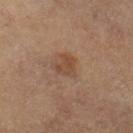{
  "biopsy_status": "not biopsied; imaged during a skin examination",
  "lesion_size": {
    "long_diameter_mm_approx": 2.5
  },
  "patient": {
    "sex": "male",
    "age_approx": 70
  },
  "image": {
    "source": "total-body photography crop",
    "field_of_view_mm": 15
  },
  "automated_metrics": {
    "area_mm2_approx": 4.5,
    "eccentricity": 0.45,
    "shape_asymmetry": 0.35,
    "border_irregularity_0_10": 3.0,
    "color_variation_0_10": 2.5,
    "peripheral_color_asymmetry": 1.0
  },
  "site": "left forearm",
  "lighting": "cross-polarized"
}